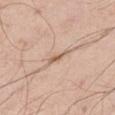| key | value |
|---|---|
| biopsy status | imaged on a skin check; not biopsied |
| size | ≈2.5 mm |
| automated metrics | a border-irregularity rating of about 3/10 and radial color variation of about 0 |
| location | the leg |
| patient | male, aged 53–57 |
| imaging modality | total-body-photography crop, ~15 mm field of view |
| illumination | white-light |